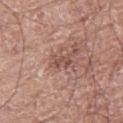The lesion was photographed on a routine skin check and not biopsied; there is no pathology result. The lesion-visualizer software estimated an area of roughly 3.5 mm². The software also gave a nevus-likeness score of about 0/100 and a lesion-detection confidence of about 95/100. This is a white-light tile. A male subject in their mid- to late 70s. A 15 mm crop from a total-body photograph taken for skin-cancer surveillance. On the right thigh.